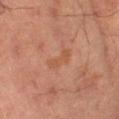Captured during whole-body skin photography for melanoma surveillance; the lesion was not biopsied.
On the left lower leg.
The patient is a male aged 68–72.
Automated image analysis of the tile measured about 5 CIELAB-L* units darker than the surrounding skin and a lesion-to-skin contrast of about 5 (normalized; higher = more distinct). And it measured a border-irregularity rating of about 4.5/10 and peripheral color asymmetry of about 0.5. The software also gave an automated nevus-likeness rating near 0 out of 100 and a lesion-detection confidence of about 100/100.
A 15 mm close-up extracted from a 3D total-body photography capture.
The tile uses cross-polarized illumination.
Measured at roughly 3 mm in maximum diameter.